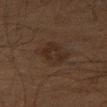Findings:
- biopsy status — catalogued during a skin exam; not biopsied
- subject — male, roughly 60 years of age
- image — ~15 mm tile from a whole-body skin photo
- lesion diameter — ~3.5 mm (longest diameter)
- site — the right thigh
- lighting — cross-polarized